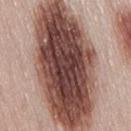{"patient": {"sex": "female", "age_approx": 50}, "image": {"source": "total-body photography crop", "field_of_view_mm": 15}, "site": "back"}